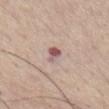Imaged during a routine full-body skin examination; the lesion was not biopsied and no histopathology is available.